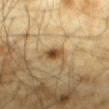The total-body-photography lesion software estimated a footprint of about 3.5 mm² and a shape eccentricity near 0.85. The analysis additionally found an average lesion color of about L≈46 a*≈16 b*≈35 (CIELAB), roughly 13 lightness units darker than nearby skin, and a lesion-to-skin contrast of about 10 (normalized; higher = more distinct). The software also gave a border-irregularity rating of about 3/10, internal color variation of about 7 on a 0–10 scale, and peripheral color asymmetry of about 2. On the mid back. A region of skin cropped from a whole-body photographic capture, roughly 15 mm wide. A male subject aged 63–67. Longest diameter approximately 2.5 mm. This is a cross-polarized tile.